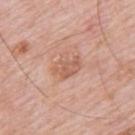Case summary:
• lesion size: ~3.5 mm (longest diameter)
• subject: male, aged 78–82
• body site: the upper back
• lighting: white-light illumination
• automated lesion analysis: a mean CIELAB color near L≈59 a*≈23 b*≈31, about 8 CIELAB-L* units darker than the surrounding skin, and a normalized lesion–skin contrast near 6; a lesion-detection confidence of about 100/100
• imaging modality: 15 mm crop, total-body photography